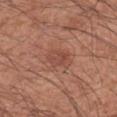biopsy_status: not biopsied; imaged during a skin examination
lesion_size:
  long_diameter_mm_approx: 3.0
lighting: white-light
site: arm
automated_metrics:
  area_mm2_approx: 6.0
  eccentricity: 0.7
  cielab_L: 48
  cielab_a: 25
  cielab_b: 28
patient:
  sex: male
  age_approx: 60
image:
  source: total-body photography crop
  field_of_view_mm: 15